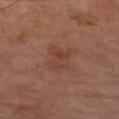This lesion was catalogued during total-body skin photography and was not selected for biopsy. Cropped from a total-body skin-imaging series; the visible field is about 15 mm. This is a cross-polarized tile. The lesion is located on the left thigh. Automated image analysis of the tile measured a border-irregularity index near 4/10 and radial color variation of about 1.5. A male patient, roughly 70 years of age.